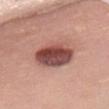Imaged during a routine full-body skin examination; the lesion was not biopsied and no histopathology is available.
Approximately 5.5 mm at its widest.
The subject is a female aged approximately 60.
Captured under white-light illumination.
An algorithmic analysis of the crop reported a lesion area of about 16 mm², a shape eccentricity near 0.8, and a shape-asymmetry score of about 0.1 (0 = symmetric). The analysis additionally found border irregularity of about 1.5 on a 0–10 scale, a within-lesion color-variation index near 8/10, and a peripheral color-asymmetry measure near 2.5. The software also gave a classifier nevus-likeness of about 85/100 and a detector confidence of about 100 out of 100 that the crop contains a lesion.
From the chest.
This image is a 15 mm lesion crop taken from a total-body photograph.